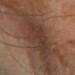{
  "biopsy_status": "not biopsied; imaged during a skin examination",
  "lighting": "cross-polarized",
  "site": "left forearm",
  "image": {
    "source": "total-body photography crop",
    "field_of_view_mm": 15
  },
  "automated_metrics": {
    "area_mm2_approx": 25.0,
    "eccentricity": 0.75,
    "shape_asymmetry": 0.25,
    "cielab_L": 33,
    "cielab_a": 17,
    "cielab_b": 24,
    "vs_skin_darker_L": 6.0,
    "vs_skin_contrast_norm": 6.0,
    "border_irregularity_0_10": 4.5,
    "peripheral_color_asymmetry": 1.0
  },
  "lesion_size": {
    "long_diameter_mm_approx": 7.5
  },
  "patient": {
    "sex": "male",
    "age_approx": 60
  }
}